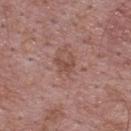Notes:
* follow-up · no biopsy performed (imaged during a skin exam)
* patient · male, approximately 70 years of age
* site · the upper back
* image · ~15 mm tile from a whole-body skin photo